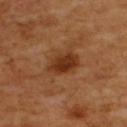Background: The patient is a male aged around 60. Automated tile analysis of the lesion measured a footprint of about 7.5 mm², an eccentricity of roughly 0.8, and a shape-asymmetry score of about 0.2 (0 = symmetric). And it measured radial color variation of about 1. The software also gave a classifier nevus-likeness of about 90/100 and a detector confidence of about 100 out of 100 that the crop contains a lesion. A 15 mm crop from a total-body photograph taken for skin-cancer surveillance. On the back. Imaged with cross-polarized lighting.